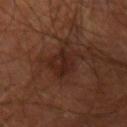Q: Is there a histopathology result?
A: imaged on a skin check; not biopsied
Q: Automated lesion metrics?
A: a mean CIELAB color near L≈23 a*≈19 b*≈23 and roughly 6 lightness units darker than nearby skin; a border-irregularity rating of about 4.5/10, internal color variation of about 3 on a 0–10 scale, and a peripheral color-asymmetry measure near 1; a nevus-likeness score of about 25/100
Q: What is the lesion's diameter?
A: ≈5 mm
Q: Lesion location?
A: the right forearm
Q: Who is the patient?
A: male, aged around 65
Q: How was this image acquired?
A: ~15 mm tile from a whole-body skin photo
Q: What lighting was used for the tile?
A: cross-polarized